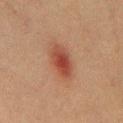Findings:
• notes · catalogued during a skin exam; not biopsied
• imaging modality · 15 mm crop, total-body photography
• patient · male, aged 38–42
• body site · the chest